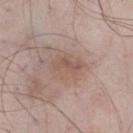Recorded during total-body skin imaging; not selected for excision or biopsy. A 15 mm close-up extracted from a 3D total-body photography capture. The lesion is on the right thigh. Automated tile analysis of the lesion measured a footprint of about 9 mm² and a shape eccentricity near 0.75. And it measured a lesion color around L≈55 a*≈17 b*≈25 in CIELAB and a normalized lesion–skin contrast near 5.5. The analysis additionally found a detector confidence of about 100 out of 100 that the crop contains a lesion. Imaged with white-light lighting. A male subject, aged around 55.